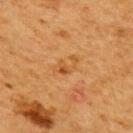No biopsy was performed on this lesion — it was imaged during a full skin examination and was not determined to be concerning. Captured under cross-polarized illumination. From the upper back. Measured at roughly 3 mm in maximum diameter. A female subject, about 50 years old. A 15 mm close-up extracted from a 3D total-body photography capture.